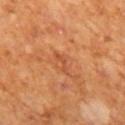acquisition: 15 mm crop, total-body photography
lighting: cross-polarized illumination
patient: male, in their mid-60s
lesion size: ≈3 mm
site: the mid back